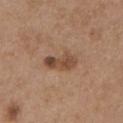Recorded during total-body skin imaging; not selected for excision or biopsy.
The patient is a female approximately 65 years of age.
Measured at roughly 4 mm in maximum diameter.
A lesion tile, about 15 mm wide, cut from a 3D total-body photograph.
The lesion is located on the chest.